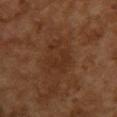– biopsy status — catalogued during a skin exam; not biopsied
– patient — female, approximately 60 years of age
– imaging modality — ~15 mm tile from a whole-body skin photo
– diameter — ~4.5 mm (longest diameter)
– location — the upper back
– illumination — cross-polarized
– TBP lesion metrics — an area of roughly 10 mm² and a shape-asymmetry score of about 0.35 (0 = symmetric); a mean CIELAB color near L≈26 a*≈17 b*≈26; border irregularity of about 5.5 on a 0–10 scale and radial color variation of about 1